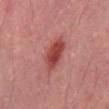Q: Was a biopsy performed?
A: total-body-photography surveillance lesion; no biopsy
Q: How was this image acquired?
A: total-body-photography crop, ~15 mm field of view
Q: What is the anatomic site?
A: the back
Q: Automated lesion metrics?
A: an average lesion color of about L≈43 a*≈29 b*≈24 (CIELAB), roughly 11 lightness units darker than nearby skin, and a lesion-to-skin contrast of about 9 (normalized; higher = more distinct); a within-lesion color-variation index near 3.5/10 and a peripheral color-asymmetry measure near 1; a nevus-likeness score of about 90/100 and a lesion-detection confidence of about 100/100
Q: Who is the patient?
A: male, in their mid- to late 40s
Q: How was the tile lit?
A: cross-polarized